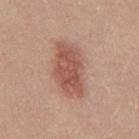<case>
  <biopsy_status>not biopsied; imaged during a skin examination</biopsy_status>
</case>